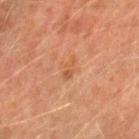biopsy status = no biopsy performed (imaged during a skin exam) | lighting = cross-polarized | automated lesion analysis = an area of roughly 3 mm² and a shape eccentricity near 0.85; a lesion–skin lightness drop of about 6; a classifier nevus-likeness of about 0/100 | diameter = ~2.5 mm (longest diameter) | image = ~15 mm tile from a whole-body skin photo | subject = male, roughly 65 years of age | body site = the left forearm.